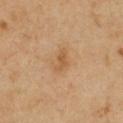Captured during whole-body skin photography for melanoma surveillance; the lesion was not biopsied. The lesion is located on the chest. Imaged with cross-polarized lighting. About 3 mm across. The total-body-photography lesion software estimated a border-irregularity rating of about 3.5/10, internal color variation of about 1 on a 0–10 scale, and a peripheral color-asymmetry measure near 0.5. The software also gave a nevus-likeness score of about 10/100. A 15 mm close-up tile from a total-body photography series done for melanoma screening. A female subject, aged approximately 40.